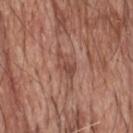notes: catalogued during a skin exam; not biopsied
image: ~15 mm tile from a whole-body skin photo
tile lighting: white-light illumination
patient: male, in their 80s
diameter: about 3 mm
automated lesion analysis: an eccentricity of roughly 0.9 and a shape-asymmetry score of about 0.4 (0 = symmetric); a detector confidence of about 60 out of 100 that the crop contains a lesion
location: the head or neck